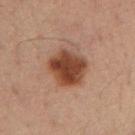Imaged during a routine full-body skin examination; the lesion was not biopsied and no histopathology is available.
An algorithmic analysis of the crop reported an eccentricity of roughly 0.6 and a symmetry-axis asymmetry near 0.2. It also reported a classifier nevus-likeness of about 100/100 and a detector confidence of about 100 out of 100 that the crop contains a lesion.
A male patient aged 33–37.
Measured at roughly 5 mm in maximum diameter.
Imaged with cross-polarized lighting.
Cropped from a total-body skin-imaging series; the visible field is about 15 mm.
On the right upper arm.